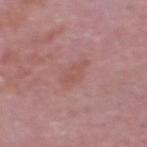The lesion was photographed on a routine skin check and not biopsied; there is no pathology result.
The subject is a male aged 63–67.
Captured under white-light illumination.
Measured at roughly 3.5 mm in maximum diameter.
The lesion is located on the head or neck.
The total-body-photography lesion software estimated internal color variation of about 2.5 on a 0–10 scale and radial color variation of about 1.
A lesion tile, about 15 mm wide, cut from a 3D total-body photograph.